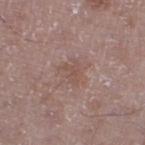Impression: No biopsy was performed on this lesion — it was imaged during a full skin examination and was not determined to be concerning. Acquisition and patient details: The subject is a male approximately 50 years of age. This image is a 15 mm lesion crop taken from a total-body photograph. Approximately 3 mm at its widest. From the right thigh. This is a white-light tile.